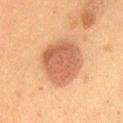Findings:
* follow-up · imaged on a skin check; not biopsied
* body site · the abdomen
* subject · female, aged 48–52
* size · ~6 mm (longest diameter)
* illumination · cross-polarized
* acquisition · ~15 mm crop, total-body skin-cancer survey